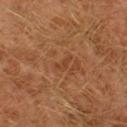Impression: Part of a total-body skin-imaging series; this lesion was reviewed on a skin check and was not flagged for biopsy. Acquisition and patient details: A male subject, about 30 years old. Cropped from a whole-body photographic skin survey; the tile spans about 15 mm. Captured under cross-polarized illumination. The lesion is located on the left thigh.